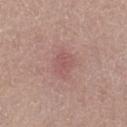Case summary:
• workup · no biopsy performed (imaged during a skin exam)
• body site · the left thigh
• subject · female, aged approximately 70
• lighting · white-light
• imaging modality · 15 mm crop, total-body photography
• size · ~3 mm (longest diameter)